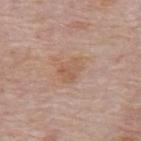workup = catalogued during a skin exam; not biopsied | subject = male, aged 73 to 77 | anatomic site = the upper back | lesion size = ≈3 mm | image = 15 mm crop, total-body photography | tile lighting = white-light illumination.